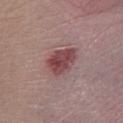site: the right lower leg; subject: male, approximately 55 years of age; image source: 15 mm crop, total-body photography.